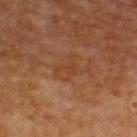{"image": {"source": "total-body photography crop", "field_of_view_mm": 15}, "site": "upper back", "automated_metrics": {"area_mm2_approx": 2.5, "eccentricity": 0.85, "vs_skin_darker_L": 5.0, "vs_skin_contrast_norm": 5.0, "lesion_detection_confidence_0_100": 100}, "lighting": "cross-polarized", "patient": {"sex": "male", "age_approx": 60}, "lesion_size": {"long_diameter_mm_approx": 2.5}}